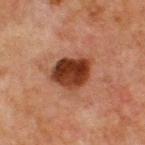This lesion was catalogued during total-body skin photography and was not selected for biopsy. From the front of the torso. A male patient aged approximately 65. The tile uses cross-polarized illumination. Cropped from a whole-body photographic skin survey; the tile spans about 15 mm. About 5 mm across. An algorithmic analysis of the crop reported internal color variation of about 6 on a 0–10 scale and radial color variation of about 2.